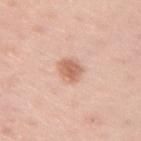Captured during whole-body skin photography for melanoma surveillance; the lesion was not biopsied. Measured at roughly 3 mm in maximum diameter. A 15 mm close-up extracted from a 3D total-body photography capture. Located on the right upper arm. The lesion-visualizer software estimated a lesion area of about 6 mm² and an eccentricity of roughly 0.65. It also reported a border-irregularity rating of about 2/10, a color-variation rating of about 2/10, and radial color variation of about 0.5. And it measured a classifier nevus-likeness of about 95/100 and lesion-presence confidence of about 100/100. A female subject aged 43 to 47.